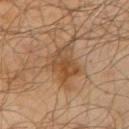Clinical impression: No biopsy was performed on this lesion — it was imaged during a full skin examination and was not determined to be concerning. Context: A 15 mm crop from a total-body photograph taken for skin-cancer surveillance. The tile uses cross-polarized illumination. The lesion is on the right upper arm. A male patient, roughly 65 years of age.